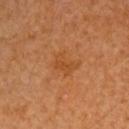No biopsy was performed on this lesion — it was imaged during a full skin examination and was not determined to be concerning.
Captured under cross-polarized illumination.
A lesion tile, about 15 mm wide, cut from a 3D total-body photograph.
Located on the arm.
Longest diameter approximately 3.5 mm.
A male patient aged around 60.